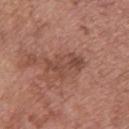Notes:
- workup · imaged on a skin check; not biopsied
- acquisition · total-body-photography crop, ~15 mm field of view
- lighting · white-light
- lesion diameter · ≈4.5 mm
- subject · female, approximately 65 years of age
- body site · the back